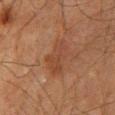Automated tile analysis of the lesion measured a mean CIELAB color near L≈35 a*≈19 b*≈27, about 6 CIELAB-L* units darker than the surrounding skin, and a lesion-to-skin contrast of about 5.5 (normalized; higher = more distinct). The analysis additionally found a color-variation rating of about 2.5/10 and peripheral color asymmetry of about 1. Located on the mid back. A male patient, aged 63 to 67. Cropped from a total-body skin-imaging series; the visible field is about 15 mm. This is a cross-polarized tile. Measured at roughly 4.5 mm in maximum diameter.